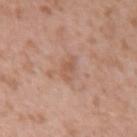Case summary:
- biopsy status: catalogued during a skin exam; not biopsied
- lesion size: ≈2.5 mm
- subject: female, aged 38–42
- location: the right upper arm
- lighting: white-light
- imaging modality: ~15 mm crop, total-body skin-cancer survey